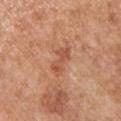Clinical impression:
This lesion was catalogued during total-body skin photography and was not selected for biopsy.